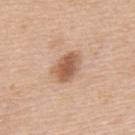Clinical impression: The lesion was tiled from a total-body skin photograph and was not biopsied. Acquisition and patient details: From the upper back. This is a white-light tile. A 15 mm close-up extracted from a 3D total-body photography capture. A male subject aged 48 to 52.